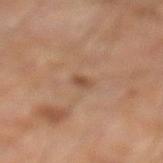{
  "automated_metrics": {
    "eccentricity": 0.75,
    "shape_asymmetry": 0.25,
    "border_irregularity_0_10": 2.5,
    "peripheral_color_asymmetry": 0.0,
    "nevus_likeness_0_100": 30,
    "lesion_detection_confidence_0_100": 100
  },
  "lesion_size": {
    "long_diameter_mm_approx": 1.5
  },
  "site": "leg",
  "patient": {
    "sex": "male",
    "age_approx": 55
  },
  "image": {
    "source": "total-body photography crop",
    "field_of_view_mm": 15
  }
}